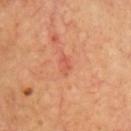Imaged during a routine full-body skin examination; the lesion was not biopsied and no histopathology is available. Imaged with cross-polarized lighting. A close-up tile cropped from a whole-body skin photograph, about 15 mm across. The subject is a male aged around 70. Located on the chest. The recorded lesion diameter is about 2.5 mm.